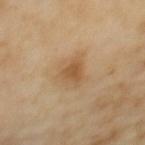No biopsy was performed on this lesion — it was imaged during a full skin examination and was not determined to be concerning. From the upper back. The subject is a female about 60 years old. Cropped from a total-body skin-imaging series; the visible field is about 15 mm.